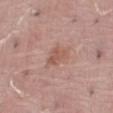| field | value |
|---|---|
| notes | imaged on a skin check; not biopsied |
| image-analysis metrics | an area of roughly 3.5 mm², an eccentricity of roughly 0.8, and a symmetry-axis asymmetry near 0.4 |
| location | the abdomen |
| acquisition | 15 mm crop, total-body photography |
| tile lighting | white-light illumination |
| diameter | ≈3 mm |
| subject | male, roughly 40 years of age |